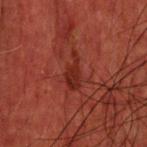Q: Was this lesion biopsied?
A: imaged on a skin check; not biopsied
Q: What is the lesion's diameter?
A: ~4 mm (longest diameter)
Q: Illumination type?
A: cross-polarized illumination
Q: What are the patient's age and sex?
A: male, about 60 years old
Q: What kind of image is this?
A: ~15 mm crop, total-body skin-cancer survey
Q: Where on the body is the lesion?
A: the head or neck